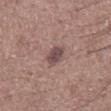Case summary:
- workup — total-body-photography surveillance lesion; no biopsy
- lesion diameter — ≈3 mm
- anatomic site — the chest
- image source — total-body-photography crop, ~15 mm field of view
- lighting — white-light
- subject — male, aged around 60